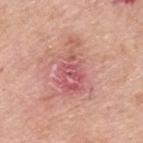* notes: total-body-photography surveillance lesion; no biopsy
* size: ~6.5 mm (longest diameter)
* image: ~15 mm tile from a whole-body skin photo
* anatomic site: the upper back
* illumination: white-light
* patient: male, approximately 70 years of age